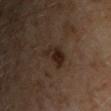Clinical summary: Located on the left upper arm. Cropped from a whole-body photographic skin survey; the tile spans about 15 mm. A male subject roughly 55 years of age.